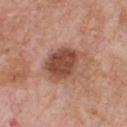<tbp_lesion>
  <biopsy_status>not biopsied; imaged during a skin examination</biopsy_status>
  <image>
    <source>total-body photography crop</source>
    <field_of_view_mm>15</field_of_view_mm>
  </image>
  <patient>
    <sex>male</sex>
    <age_approx>60</age_approx>
  </patient>
  <site>chest</site>
  <lighting>white-light</lighting>
</tbp_lesion>